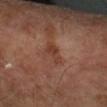<tbp_lesion>
<biopsy_status>not biopsied; imaged during a skin examination</biopsy_status>
<lesion_size>
  <long_diameter_mm_approx>3.5</long_diameter_mm_approx>
</lesion_size>
<automated_metrics>
  <cielab_L>38</cielab_L>
  <cielab_a>23</cielab_a>
  <cielab_b>28</cielab_b>
  <vs_skin_darker_L>8.0</vs_skin_darker_L>
  <vs_skin_contrast_norm>7.0</vs_skin_contrast_norm>
  <border_irregularity_0_10>3.5</border_irregularity_0_10>
  <color_variation_0_10>1.5</color_variation_0_10>
  <peripheral_color_asymmetry>0.5</peripheral_color_asymmetry>
  <lesion_detection_confidence_0_100>100</lesion_detection_confidence_0_100>
</automated_metrics>
<image>
  <source>total-body photography crop</source>
  <field_of_view_mm>15</field_of_view_mm>
</image>
<patient>
  <age_approx>65</age_approx>
</patient>
<lighting>cross-polarized</lighting>
<site>right lower leg</site>
</tbp_lesion>